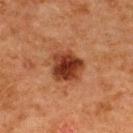Impression:
The lesion was tiled from a total-body skin photograph and was not biopsied.
Acquisition and patient details:
Located on the upper back. Captured under cross-polarized illumination. Cropped from a whole-body photographic skin survey; the tile spans about 15 mm. A female subject, approximately 50 years of age. Automated image analysis of the tile measured a lesion area of about 12 mm² and an eccentricity of roughly 0.35. It also reported a lesion color around L≈33 a*≈24 b*≈30 in CIELAB and a normalized border contrast of about 11.5. It also reported an automated nevus-likeness rating near 95 out of 100. Longest diameter approximately 4 mm.